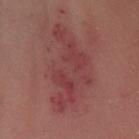Part of a total-body skin-imaging series; this lesion was reviewed on a skin check and was not flagged for biopsy. An algorithmic analysis of the crop reported an average lesion color of about L≈42 a*≈28 b*≈21 (CIELAB) and about 7 CIELAB-L* units darker than the surrounding skin. And it measured a border-irregularity index near 7/10, a within-lesion color-variation index near 5/10, and a peripheral color-asymmetry measure near 2. Cropped from a total-body skin-imaging series; the visible field is about 15 mm. A female patient, aged 38 to 42. The lesion's longest dimension is about 10 mm. From the leg. Imaged with cross-polarized lighting.